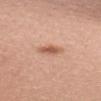Impression: No biopsy was performed on this lesion — it was imaged during a full skin examination and was not determined to be concerning. Acquisition and patient details: The lesion's longest dimension is about 2.5 mm. The lesion is located on the upper back. The lesion-visualizer software estimated a lesion area of about 4 mm², a shape eccentricity near 0.8, and two-axis asymmetry of about 0.25. The analysis additionally found a detector confidence of about 100 out of 100 that the crop contains a lesion. A close-up tile cropped from a whole-body skin photograph, about 15 mm across. The patient is a female in their mid-30s. Captured under white-light illumination.